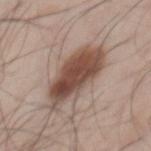Recorded during total-body skin imaging; not selected for excision or biopsy. On the mid back. A male subject in their mid-40s. A 15 mm crop from a total-body photograph taken for skin-cancer surveillance. The tile uses white-light illumination. Automated tile analysis of the lesion measured a footprint of about 22 mm², a shape eccentricity near 0.85, and a symmetry-axis asymmetry near 0.2. And it measured a border-irregularity index near 3/10, a color-variation rating of about 5.5/10, and radial color variation of about 1.5. The software also gave a nevus-likeness score of about 95/100.